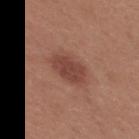Acquisition and patient details: The lesion is on the upper back. Imaged with white-light lighting. The patient is a female approximately 25 years of age. This image is a 15 mm lesion crop taken from a total-body photograph.